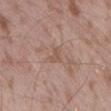The lesion was photographed on a routine skin check and not biopsied; there is no pathology result. Automated tile analysis of the lesion measured an area of roughly 2.5 mm², a shape eccentricity near 0.9, and two-axis asymmetry of about 0.4. A 15 mm close-up tile from a total-body photography series done for melanoma screening. On the back. The recorded lesion diameter is about 2.5 mm. The subject is a male aged approximately 55. The tile uses white-light illumination.